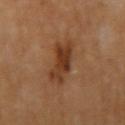Recorded during total-body skin imaging; not selected for excision or biopsy. A female patient approximately 55 years of age. A roughly 15 mm field-of-view crop from a total-body skin photograph. About 5.5 mm across. The lesion-visualizer software estimated an area of roughly 11 mm², a shape eccentricity near 0.9, and a symmetry-axis asymmetry near 0.35. From the right upper arm.